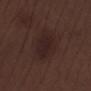Q: Was a biopsy performed?
A: no biopsy performed (imaged during a skin exam)
Q: Illumination type?
A: white-light
Q: Where on the body is the lesion?
A: the right lower leg
Q: What is the lesion's diameter?
A: about 5.5 mm
Q: What kind of image is this?
A: ~15 mm tile from a whole-body skin photo
Q: What are the patient's age and sex?
A: male, aged around 70